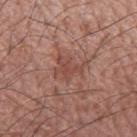Part of a total-body skin-imaging series; this lesion was reviewed on a skin check and was not flagged for biopsy.
A close-up tile cropped from a whole-body skin photograph, about 15 mm across.
Longest diameter approximately 3 mm.
The lesion is located on the left forearm.
A male patient approximately 55 years of age.